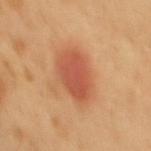No biopsy was performed on this lesion — it was imaged during a full skin examination and was not determined to be concerning.
Cropped from a whole-body photographic skin survey; the tile spans about 15 mm.
Measured at roughly 5.5 mm in maximum diameter.
The lesion is located on the mid back.
The tile uses cross-polarized illumination.
A male patient, about 50 years old.
An algorithmic analysis of the crop reported a lesion area of about 16 mm², an eccentricity of roughly 0.75, and two-axis asymmetry of about 0.15. And it measured a border-irregularity rating of about 1.5/10, internal color variation of about 4 on a 0–10 scale, and peripheral color asymmetry of about 1. And it measured lesion-presence confidence of about 100/100.